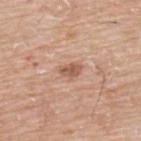The lesion was photographed on a routine skin check and not biopsied; there is no pathology result.
The total-body-photography lesion software estimated a footprint of about 3.5 mm² and a symmetry-axis asymmetry near 0.2. It also reported a lesion color around L≈57 a*≈22 b*≈29 in CIELAB and roughly 11 lightness units darker than nearby skin.
The subject is a male about 75 years old.
The lesion is located on the upper back.
A region of skin cropped from a whole-body photographic capture, roughly 15 mm wide.
The recorded lesion diameter is about 2.5 mm.